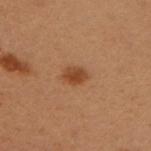Captured during whole-body skin photography for melanoma surveillance; the lesion was not biopsied.
The lesion is located on the left upper arm.
Approximately 2.5 mm at its widest.
The subject is a female in their 50s.
A lesion tile, about 15 mm wide, cut from a 3D total-body photograph.
Imaged with cross-polarized lighting.